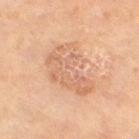<record>
  <biopsy_status>not biopsied; imaged during a skin examination</biopsy_status>
  <lesion_size>
    <long_diameter_mm_approx>6.0</long_diameter_mm_approx>
  </lesion_size>
  <patient>
    <sex>male</sex>
    <age_approx>65</age_approx>
  </patient>
  <site>leg</site>
  <image>
    <source>total-body photography crop</source>
    <field_of_view_mm>15</field_of_view_mm>
  </image>
  <lighting>cross-polarized</lighting>
</record>